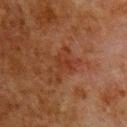Notes:
* workup · imaged on a skin check; not biopsied
* image source · ~15 mm crop, total-body skin-cancer survey
* anatomic site · the upper back
* image-analysis metrics · an area of roughly 6 mm², a shape eccentricity near 0.85, and a shape-asymmetry score of about 0.4 (0 = symmetric); a border-irregularity rating of about 7/10, a within-lesion color-variation index near 2.5/10, and radial color variation of about 1; an automated nevus-likeness rating near 0 out of 100
* patient · male, aged around 80
* lighting · cross-polarized
* diameter · ≈4.5 mm